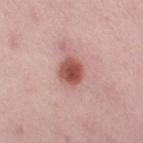Part of a total-body skin-imaging series; this lesion was reviewed on a skin check and was not flagged for biopsy. A close-up tile cropped from a whole-body skin photograph, about 15 mm across. On the right thigh. A female patient in their 30s.